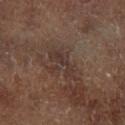subject: male, aged around 65; body site: the left lower leg; imaging modality: ~15 mm tile from a whole-body skin photo.